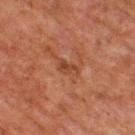follow-up = catalogued during a skin exam; not biopsied | diameter = ≈2.5 mm | patient = male, approximately 60 years of age | acquisition = total-body-photography crop, ~15 mm field of view | site = the upper back.